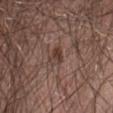Impression: Part of a total-body skin-imaging series; this lesion was reviewed on a skin check and was not flagged for biopsy. Background: The subject is a male approximately 55 years of age. Approximately 2.5 mm at its widest. A 15 mm close-up tile from a total-body photography series done for melanoma screening. The lesion is located on the abdomen. This is a white-light tile.